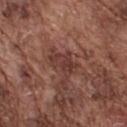Clinical impression:
No biopsy was performed on this lesion — it was imaged during a full skin examination and was not determined to be concerning.
Image and clinical context:
The lesion is on the back. Measured at roughly 3.5 mm in maximum diameter. A 15 mm close-up extracted from a 3D total-body photography capture. This is a white-light tile. A male patient aged around 75.